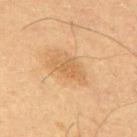– follow-up — no biopsy performed (imaged during a skin exam)
– image-analysis metrics — a mean CIELAB color near L≈54 a*≈18 b*≈37 and roughly 7 lightness units darker than nearby skin; a color-variation rating of about 1.5/10 and radial color variation of about 0.5; a detector confidence of about 100 out of 100 that the crop contains a lesion
– subject — male, approximately 60 years of age
– image — total-body-photography crop, ~15 mm field of view
– lesion diameter — ~3 mm (longest diameter)